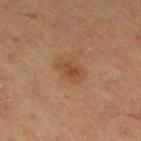Clinical impression: No biopsy was performed on this lesion — it was imaged during a full skin examination and was not determined to be concerning. Clinical summary: Captured under cross-polarized illumination. A female patient roughly 60 years of age. Measured at roughly 3.5 mm in maximum diameter. The lesion is on the leg. A 15 mm close-up extracted from a 3D total-body photography capture.